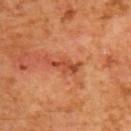| key | value |
|---|---|
| follow-up | total-body-photography surveillance lesion; no biopsy |
| site | the upper back |
| patient | male, aged around 65 |
| lighting | cross-polarized |
| lesion size | about 4 mm |
| automated metrics | a footprint of about 4 mm² and two-axis asymmetry of about 0.45; an average lesion color of about L≈47 a*≈32 b*≈38 (CIELAB), roughly 10 lightness units darker than nearby skin, and a normalized lesion–skin contrast near 7.5; a border-irregularity index near 6/10, a color-variation rating of about 0/10, and a peripheral color-asymmetry measure near 0 |
| acquisition | total-body-photography crop, ~15 mm field of view |